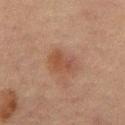Imaged during a routine full-body skin examination; the lesion was not biopsied and no histopathology is available.